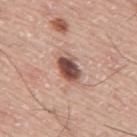Clinical impression: Recorded during total-body skin imaging; not selected for excision or biopsy. Background: A male patient in their mid-70s. About 4 mm across. From the back. A 15 mm close-up tile from a total-body photography series done for melanoma screening. This is a white-light tile.